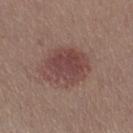No biopsy was performed on this lesion — it was imaged during a full skin examination and was not determined to be concerning.
Located on the right thigh.
The lesion's longest dimension is about 5.5 mm.
The subject is a female roughly 40 years of age.
A lesion tile, about 15 mm wide, cut from a 3D total-body photograph.